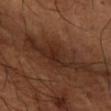notes=no biopsy performed (imaged during a skin exam)
image=15 mm crop, total-body photography
illumination=cross-polarized
site=the right forearm
TBP lesion metrics=an average lesion color of about L≈27 a*≈19 b*≈26 (CIELAB) and roughly 8 lightness units darker than nearby skin
subject=male, aged 63 to 67